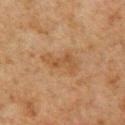Recorded during total-body skin imaging; not selected for excision or biopsy. The lesion's longest dimension is about 4.5 mm. From the chest. A 15 mm close-up tile from a total-body photography series done for melanoma screening. A male patient aged 58 to 62.